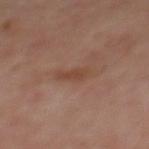Imaged during a routine full-body skin examination; the lesion was not biopsied and no histopathology is available. A male subject approximately 50 years of age. The lesion is located on the back. A 15 mm close-up tile from a total-body photography series done for melanoma screening. Measured at roughly 3.5 mm in maximum diameter.